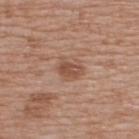Q: Was a biopsy performed?
A: catalogued during a skin exam; not biopsied
Q: What kind of image is this?
A: total-body-photography crop, ~15 mm field of view
Q: Patient demographics?
A: male, in their mid- to late 60s
Q: Where on the body is the lesion?
A: the upper back
Q: What lighting was used for the tile?
A: white-light illumination